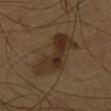Assessment:
Part of a total-body skin-imaging series; this lesion was reviewed on a skin check and was not flagged for biopsy.
Clinical summary:
Imaged with cross-polarized lighting. A male patient, aged around 60. On the chest. An algorithmic analysis of the crop reported a border-irregularity index near 4.5/10, a color-variation rating of about 5/10, and a peripheral color-asymmetry measure near 2. This image is a 15 mm lesion crop taken from a total-body photograph. The lesion's longest dimension is about 6.5 mm.